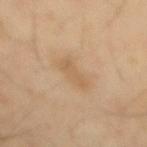biopsy status: no biopsy performed (imaged during a skin exam)